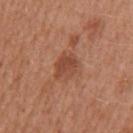– biopsy status: imaged on a skin check; not biopsied
– illumination: white-light illumination
– patient: female, approximately 40 years of age
– automated lesion analysis: a lesion area of about 6 mm², an eccentricity of roughly 0.65, and a shape-asymmetry score of about 0.3 (0 = symmetric); a border-irregularity index near 3/10 and internal color variation of about 2.5 on a 0–10 scale; an automated nevus-likeness rating near 5 out of 100
– anatomic site: the left upper arm
– image: total-body-photography crop, ~15 mm field of view
– diameter: about 3.5 mm